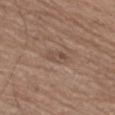Q: Was this lesion biopsied?
A: imaged on a skin check; not biopsied
Q: Automated lesion metrics?
A: a mean CIELAB color near L≈48 a*≈17 b*≈25, about 7 CIELAB-L* units darker than the surrounding skin, and a normalized lesion–skin contrast near 5; an automated nevus-likeness rating near 0 out of 100 and a lesion-detection confidence of about 95/100
Q: What is the anatomic site?
A: the left thigh
Q: Illumination type?
A: white-light
Q: Lesion size?
A: ~3 mm (longest diameter)
Q: What are the patient's age and sex?
A: male, roughly 70 years of age
Q: What is the imaging modality?
A: total-body-photography crop, ~15 mm field of view